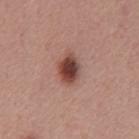Q: Was this lesion biopsied?
A: total-body-photography surveillance lesion; no biopsy
Q: What are the patient's age and sex?
A: male, in their mid-50s
Q: How was this image acquired?
A: total-body-photography crop, ~15 mm field of view
Q: Where on the body is the lesion?
A: the mid back